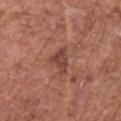No biopsy was performed on this lesion — it was imaged during a full skin examination and was not determined to be concerning. The lesion is on the front of the torso. Captured under white-light illumination. Automated tile analysis of the lesion measured an area of roughly 5 mm², an eccentricity of roughly 0.7, and a shape-asymmetry score of about 0.45 (0 = symmetric). Longest diameter approximately 3.5 mm. A roughly 15 mm field-of-view crop from a total-body skin photograph. A male subject, aged around 75.